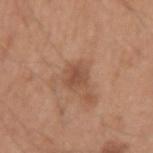Q: Lesion location?
A: the left upper arm
Q: What is the lesion's diameter?
A: ≈3 mm
Q: Who is the patient?
A: male, about 50 years old
Q: What did automated image analysis measure?
A: an area of roughly 5.5 mm², a shape eccentricity near 0.45, and two-axis asymmetry of about 0.2; a lesion color around L≈50 a*≈21 b*≈31 in CIELAB and about 8 CIELAB-L* units darker than the surrounding skin
Q: What kind of image is this?
A: total-body-photography crop, ~15 mm field of view